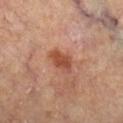Imaged with cross-polarized lighting.
The total-body-photography lesion software estimated border irregularity of about 2.5 on a 0–10 scale, internal color variation of about 3.5 on a 0–10 scale, and peripheral color asymmetry of about 1.
The lesion is on the right lower leg.
A male patient in their mid- to late 60s.
Approximately 3.5 mm at its widest.
This image is a 15 mm lesion crop taken from a total-body photograph.